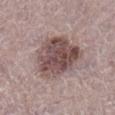image-analysis metrics = a mean CIELAB color near L≈48 a*≈17 b*≈19, a lesion–skin lightness drop of about 14, and a normalized border contrast of about 10; acquisition = total-body-photography crop, ~15 mm field of view; subject = female, about 65 years old; body site = the right lower leg; diameter = ≈6.5 mm.